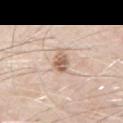The lesion was photographed on a routine skin check and not biopsied; there is no pathology result. A male subject, about 70 years old. Measured at roughly 3 mm in maximum diameter. Automated image analysis of the tile measured a normalized lesion–skin contrast near 8. This image is a 15 mm lesion crop taken from a total-body photograph. The tile uses white-light illumination. The lesion is on the left upper arm.